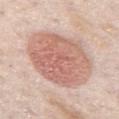Q: Is there a histopathology result?
A: catalogued during a skin exam; not biopsied
Q: Lesion location?
A: the mid back
Q: Illumination type?
A: white-light
Q: What are the patient's age and sex?
A: male, aged 73 to 77
Q: What is the imaging modality?
A: total-body-photography crop, ~15 mm field of view